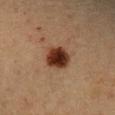| field | value |
|---|---|
| biopsy status | no biopsy performed (imaged during a skin exam) |
| size | about 3.5 mm |
| image-analysis metrics | an area of roughly 8.5 mm², an outline eccentricity of about 0.35 (0 = round, 1 = elongated), and a symmetry-axis asymmetry near 0.1; a border-irregularity index near 1/10, a within-lesion color-variation index near 5/10, and peripheral color asymmetry of about 1.5; an automated nevus-likeness rating near 100 out of 100 |
| image source | total-body-photography crop, ~15 mm field of view |
| subject | female, aged approximately 45 |
| anatomic site | the right forearm |
| lighting | cross-polarized |